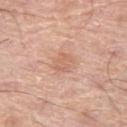Captured during whole-body skin photography for melanoma surveillance; the lesion was not biopsied.
Approximately 2.5 mm at its widest.
The subject is a male aged 78–82.
From the left thigh.
A lesion tile, about 15 mm wide, cut from a 3D total-body photograph.
The lesion-visualizer software estimated an area of roughly 5 mm² and an eccentricity of roughly 0.45. The analysis additionally found a lesion-detection confidence of about 100/100.